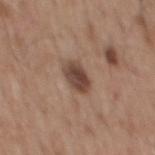image source: total-body-photography crop, ~15 mm field of view
automated lesion analysis: an average lesion color of about L≈44 a*≈18 b*≈25 (CIELAB), a lesion–skin lightness drop of about 13, and a normalized border contrast of about 10; border irregularity of about 1.5 on a 0–10 scale, a within-lesion color-variation index near 4/10, and a peripheral color-asymmetry measure near 1; a classifier nevus-likeness of about 70/100
location: the mid back
lighting: white-light illumination
subject: male, in their mid- to late 50s